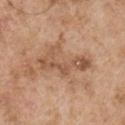Impression: The lesion was tiled from a total-body skin photograph and was not biopsied. Image and clinical context: A 15 mm close-up tile from a total-body photography series done for melanoma screening. This is a white-light tile. The patient is a male in their mid- to late 50s. An algorithmic analysis of the crop reported an outline eccentricity of about 0.9 (0 = round, 1 = elongated) and a symmetry-axis asymmetry near 0.7. Longest diameter approximately 6.5 mm. The lesion is located on the right upper arm.